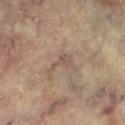Part of a total-body skin-imaging series; this lesion was reviewed on a skin check and was not flagged for biopsy. A roughly 15 mm field-of-view crop from a total-body skin photograph. A female subject, about 80 years old. Imaged with cross-polarized lighting. The lesion is on the left lower leg. Longest diameter approximately 3.5 mm.